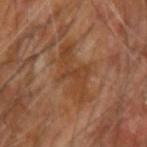workup: imaged on a skin check; not biopsied
image: ~15 mm tile from a whole-body skin photo
anatomic site: the right forearm
lighting: cross-polarized illumination
automated lesion analysis: a footprint of about 16 mm², an outline eccentricity of about 0.85 (0 = round, 1 = elongated), and a symmetry-axis asymmetry near 0.35; a mean CIELAB color near L≈43 a*≈22 b*≈34, about 6 CIELAB-L* units darker than the surrounding skin, and a normalized border contrast of about 6
patient: male, roughly 65 years of age